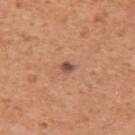notes: no biopsy performed (imaged during a skin exam) | tile lighting: white-light | size: about 1.5 mm | patient: male, approximately 55 years of age | imaging modality: ~15 mm crop, total-body skin-cancer survey | site: the arm | image-analysis metrics: a lesion area of about 2 mm², an outline eccentricity of about 0.55 (0 = round, 1 = elongated), and two-axis asymmetry of about 0.35; a border-irregularity index near 2.5/10, internal color variation of about 1 on a 0–10 scale, and peripheral color asymmetry of about 0.